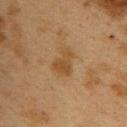Recorded during total-body skin imaging; not selected for excision or biopsy.
Cropped from a total-body skin-imaging series; the visible field is about 15 mm.
The lesion's longest dimension is about 3 mm.
A female patient, aged approximately 40.
On the upper back.
This is a cross-polarized tile.